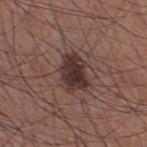Assessment: Recorded during total-body skin imaging; not selected for excision or biopsy. Clinical summary: From the upper back. The total-body-photography lesion software estimated an area of roughly 10 mm², an outline eccentricity of about 0.75 (0 = round, 1 = elongated), and two-axis asymmetry of about 0.2. It also reported an average lesion color of about L≈33 a*≈17 b*≈18 (CIELAB), a lesion–skin lightness drop of about 13, and a normalized lesion–skin contrast near 11.5. The software also gave a border-irregularity rating of about 2.5/10, a color-variation rating of about 4.5/10, and radial color variation of about 1.5. Captured under white-light illumination. A male subject, aged 33–37. A roughly 15 mm field-of-view crop from a total-body skin photograph. Longest diameter approximately 4 mm.